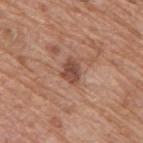Findings:
• follow-up: total-body-photography surveillance lesion; no biopsy
• patient: male, aged around 65
• diameter: ~3 mm (longest diameter)
• automated lesion analysis: a footprint of about 5 mm² and a shape-asymmetry score of about 0.3 (0 = symmetric); a lesion color around L≈48 a*≈22 b*≈28 in CIELAB, a lesion–skin lightness drop of about 11, and a lesion-to-skin contrast of about 8 (normalized; higher = more distinct); peripheral color asymmetry of about 1; a classifier nevus-likeness of about 5/100 and a lesion-detection confidence of about 100/100
• lighting: white-light illumination
• location: the upper back
• acquisition: ~15 mm crop, total-body skin-cancer survey